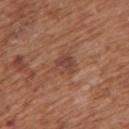follow-up: imaged on a skin check; not biopsied | image-analysis metrics: an eccentricity of roughly 0.6 and a symmetry-axis asymmetry near 0.3; a nevus-likeness score of about 0/100 and a detector confidence of about 100 out of 100 that the crop contains a lesion | subject: female, aged 73–77 | acquisition: ~15 mm crop, total-body skin-cancer survey | diameter: about 3 mm | site: the upper back.